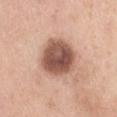Impression: Recorded during total-body skin imaging; not selected for excision or biopsy. Image and clinical context: Approximately 5.5 mm at its widest. From the right thigh. Captured under white-light illumination. A female patient about 55 years old. A 15 mm close-up tile from a total-body photography series done for melanoma screening. The total-body-photography lesion software estimated a lesion color around L≈54 a*≈21 b*≈27 in CIELAB and a lesion–skin lightness drop of about 18.